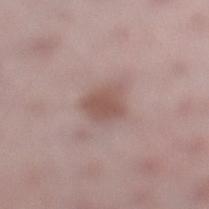Imaged during a routine full-body skin examination; the lesion was not biopsied and no histopathology is available. Automated image analysis of the tile measured a lesion–skin lightness drop of about 10 and a lesion-to-skin contrast of about 7 (normalized; higher = more distinct). The software also gave a nevus-likeness score of about 60/100. A female patient about 45 years old. A 15 mm crop from a total-body photograph taken for skin-cancer surveillance. Located on the left lower leg. Captured under white-light illumination.